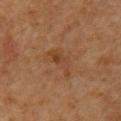Findings:
• notes: no biopsy performed (imaged during a skin exam)
• lighting: cross-polarized
• imaging modality: 15 mm crop, total-body photography
• body site: the chest
• subject: male, in their 60s
• lesion size: ≈4 mm